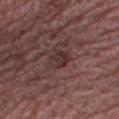workup = catalogued during a skin exam; not biopsied | body site = the leg | subject = male, aged around 65 | image = ~15 mm crop, total-body skin-cancer survey | lighting = white-light illumination.